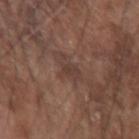workup: total-body-photography surveillance lesion; no biopsy
illumination: white-light illumination
lesion diameter: ~3.5 mm (longest diameter)
image source: 15 mm crop, total-body photography
body site: the left forearm
patient: male, aged 68–72
automated lesion analysis: a footprint of about 4.5 mm², an eccentricity of roughly 0.75, and a symmetry-axis asymmetry near 0.45; a border-irregularity index near 5/10 and a peripheral color-asymmetry measure near 0.5; a nevus-likeness score of about 0/100 and lesion-presence confidence of about 80/100